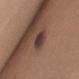<record>
<biopsy_status>not biopsied; imaged during a skin examination</biopsy_status>
<site>chest</site>
<lighting>white-light</lighting>
<automated_metrics>
  <eccentricity>0.6</eccentricity>
  <shape_asymmetry>0.2</shape_asymmetry>
  <cielab_L>37</cielab_L>
  <cielab_a>17</cielab_a>
  <cielab_b>20</cielab_b>
  <vs_skin_contrast_norm>11.5</vs_skin_contrast_norm>
  <peripheral_color_asymmetry>1.5</peripheral_color_asymmetry>
</automated_metrics>
<lesion_size>
  <long_diameter_mm_approx>3.0</long_diameter_mm_approx>
</lesion_size>
<image>
  <source>total-body photography crop</source>
  <field_of_view_mm>15</field_of_view_mm>
</image>
<patient>
  <sex>female</sex>
  <age_approx>20</age_approx>
</patient>
</record>